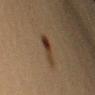A female patient, aged 38–42. On the left upper arm. Cropped from a whole-body photographic skin survey; the tile spans about 15 mm. Approximately 3 mm at its widest.